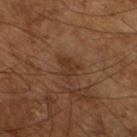site: left lower leg
lighting: cross-polarized
patient:
  sex: male
  age_approx: 65
image:
  source: total-body photography crop
  field_of_view_mm: 15
automated_metrics:
  area_mm2_approx: 5.5
  eccentricity: 0.65
  shape_asymmetry: 0.5
  cielab_L: 33
  cielab_a: 18
  cielab_b: 28
  vs_skin_darker_L: 6.0
  vs_skin_contrast_norm: 6.0
  border_irregularity_0_10: 5.5
  color_variation_0_10: 2.0
  peripheral_color_asymmetry: 0.5
  nevus_likeness_0_100: 0
  lesion_detection_confidence_0_100: 100